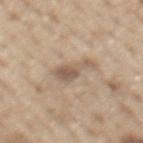This lesion was catalogued during total-body skin photography and was not selected for biopsy. Approximately 4 mm at its widest. Automated tile analysis of the lesion measured border irregularity of about 6 on a 0–10 scale, internal color variation of about 2.5 on a 0–10 scale, and radial color variation of about 1. The analysis additionally found an automated nevus-likeness rating near 0 out of 100. Cropped from a total-body skin-imaging series; the visible field is about 15 mm. The tile uses white-light illumination. A male subject in their 70s. The lesion is located on the back.